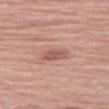<lesion>
<biopsy_status>not biopsied; imaged during a skin examination</biopsy_status>
</lesion>